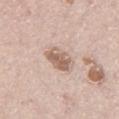biopsy status = no biopsy performed (imaged during a skin exam); image = ~15 mm tile from a whole-body skin photo; anatomic site = the left thigh; lesion diameter = about 4 mm; image-analysis metrics = internal color variation of about 4 on a 0–10 scale and a peripheral color-asymmetry measure near 1.5; patient = male, aged 63 to 67; tile lighting = white-light illumination.